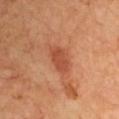{
  "biopsy_status": "not biopsied; imaged during a skin examination",
  "image": {
    "source": "total-body photography crop",
    "field_of_view_mm": 15
  },
  "patient": {
    "sex": "male",
    "age_approx": 70
  },
  "automated_metrics": {
    "vs_skin_contrast_norm": 7.0,
    "border_irregularity_0_10": 2.0,
    "color_variation_0_10": 2.0,
    "peripheral_color_asymmetry": 0.5
  },
  "lesion_size": {
    "long_diameter_mm_approx": 3.5
  },
  "site": "left upper arm"
}